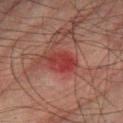Captured during whole-body skin photography for melanoma surveillance; the lesion was not biopsied. A 15 mm crop from a total-body photograph taken for skin-cancer surveillance. This is a cross-polarized tile. The patient is a male in their mid- to late 70s. Located on the leg.